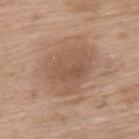Case summary:
• workup — imaged on a skin check; not biopsied